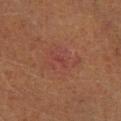notes: total-body-photography surveillance lesion; no biopsy | anatomic site: the right lower leg | image: 15 mm crop, total-body photography | patient: female, aged approximately 40.